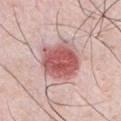Assessment: This lesion was catalogued during total-body skin photography and was not selected for biopsy. Clinical summary: A close-up tile cropped from a whole-body skin photograph, about 15 mm across. A male patient, aged around 35. This is a white-light tile. About 5.5 mm across. The lesion-visualizer software estimated a footprint of about 18 mm² and two-axis asymmetry of about 0.15. The software also gave an average lesion color of about L≈57 a*≈28 b*≈23 (CIELAB), about 16 CIELAB-L* units darker than the surrounding skin, and a lesion-to-skin contrast of about 10 (normalized; higher = more distinct). The lesion is located on the chest.